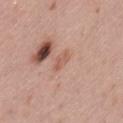Assessment:
Imaged during a routine full-body skin examination; the lesion was not biopsied and no histopathology is available.
Context:
This is a white-light tile. A lesion tile, about 15 mm wide, cut from a 3D total-body photograph. A female patient aged approximately 30. From the left thigh. Approximately 2.5 mm at its widest.